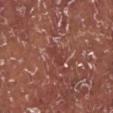Notes:
• notes — imaged on a skin check; not biopsied
• patient — male, in their mid-50s
• anatomic site — the left lower leg
• lesion diameter — ≈2.5 mm
• illumination — white-light illumination
• imaging modality — 15 mm crop, total-body photography